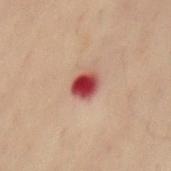Case summary:
- biopsy status: total-body-photography surveillance lesion; no biopsy
- TBP lesion metrics: a mean CIELAB color near L≈37 a*≈30 b*≈23, a lesion–skin lightness drop of about 17, and a normalized border contrast of about 13.5; a nevus-likeness score of about 0/100 and a detector confidence of about 100 out of 100 that the crop contains a lesion
- tile lighting: cross-polarized illumination
- lesion diameter: about 2.5 mm
- subject: male, in their 50s
- acquisition: ~15 mm crop, total-body skin-cancer survey
- location: the mid back